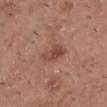Impression:
The lesion was photographed on a routine skin check and not biopsied; there is no pathology result.
Context:
The lesion's longest dimension is about 3 mm. A 15 mm close-up tile from a total-body photography series done for melanoma screening. Captured under white-light illumination. Located on the head or neck. The lesion-visualizer software estimated an average lesion color of about L≈46 a*≈22 b*≈28 (CIELAB), roughly 9 lightness units darker than nearby skin, and a normalized border contrast of about 7. And it measured border irregularity of about 2 on a 0–10 scale, a color-variation rating of about 4/10, and a peripheral color-asymmetry measure near 1.5. The analysis additionally found a nevus-likeness score of about 10/100 and lesion-presence confidence of about 100/100. A male subject in their mid- to late 50s.